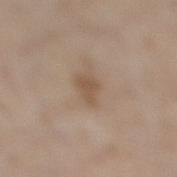biopsy_status: not biopsied; imaged during a skin examination
patient:
  sex: male
  age_approx: 60
lighting: white-light
lesion_size:
  long_diameter_mm_approx: 2.5
automated_metrics:
  area_mm2_approx: 4.5
  shape_asymmetry: 0.35
  vs_skin_contrast_norm: 6.0
  border_irregularity_0_10: 3.0
  color_variation_0_10: 1.5
image:
  source: total-body photography crop
  field_of_view_mm: 15
site: leg